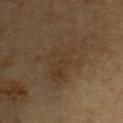Case summary:
• notes · no biopsy performed (imaged during a skin exam)
• lesion diameter · about 7 mm
• TBP lesion metrics · border irregularity of about 6.5 on a 0–10 scale, a within-lesion color-variation index near 3/10, and a peripheral color-asymmetry measure near 1; a classifier nevus-likeness of about 0/100 and a detector confidence of about 100 out of 100 that the crop contains a lesion
• site · the chest
• illumination · cross-polarized
• acquisition · ~15 mm tile from a whole-body skin photo
• patient · male, aged around 85 or older